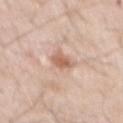Imaged during a routine full-body skin examination; the lesion was not biopsied and no histopathology is available. Automated tile analysis of the lesion measured a lesion area of about 4 mm², an outline eccentricity of about 0.75 (0 = round, 1 = elongated), and a shape-asymmetry score of about 0.2 (0 = symmetric). And it measured a lesion color around L≈62 a*≈21 b*≈30 in CIELAB, roughly 12 lightness units darker than nearby skin, and a normalized border contrast of about 7.5. The analysis additionally found an automated nevus-likeness rating near 25 out of 100 and lesion-presence confidence of about 100/100. The lesion is on the mid back. The lesion's longest dimension is about 3 mm. A 15 mm crop from a total-body photograph taken for skin-cancer surveillance. The patient is a male aged 58 to 62. Captured under white-light illumination.